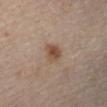follow-up = catalogued during a skin exam; not biopsied
size = about 2.5 mm
acquisition = total-body-photography crop, ~15 mm field of view
patient = male, approximately 50 years of age
lighting = cross-polarized
location = the leg